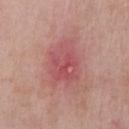Findings:
- biopsy status · total-body-photography surveillance lesion; no biopsy
- anatomic site · the chest
- subject · male, roughly 60 years of age
- diameter · about 6 mm
- acquisition · ~15 mm tile from a whole-body skin photo
- illumination · white-light illumination